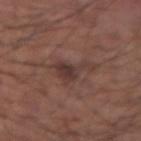Q: Was this lesion biopsied?
A: total-body-photography surveillance lesion; no biopsy
Q: How was this image acquired?
A: total-body-photography crop, ~15 mm field of view
Q: Illumination type?
A: white-light illumination
Q: Who is the patient?
A: male, in their mid-50s
Q: How large is the lesion?
A: ≈5 mm
Q: What is the anatomic site?
A: the left forearm